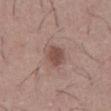{
  "biopsy_status": "not biopsied; imaged during a skin examination",
  "lighting": "white-light",
  "patient": {
    "sex": "male",
    "age_approx": 45
  },
  "lesion_size": {
    "long_diameter_mm_approx": 3.0
  },
  "site": "front of the torso",
  "image": {
    "source": "total-body photography crop",
    "field_of_view_mm": 15
  }
}